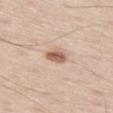Assessment:
The lesion was tiled from a total-body skin photograph and was not biopsied.
Context:
A male patient aged 58–62. A roughly 15 mm field-of-view crop from a total-body skin photograph. Longest diameter approximately 2.5 mm. Located on the left thigh. The total-body-photography lesion software estimated an average lesion color of about L≈59 a*≈19 b*≈29 (CIELAB) and a normalized lesion–skin contrast near 9. It also reported an automated nevus-likeness rating near 95 out of 100.